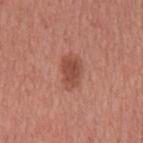notes=total-body-photography surveillance lesion; no biopsy.